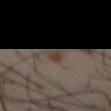This image is a 15 mm lesion crop taken from a total-body photograph. This is a cross-polarized tile. A male subject, about 40 years old. The lesion's longest dimension is about 3 mm. The lesion is on the abdomen.